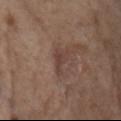Case summary:
* subject: female, roughly 85 years of age or older
* acquisition: ~15 mm tile from a whole-body skin photo
* body site: the front of the torso